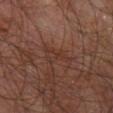Image and clinical context: This image is a 15 mm lesion crop taken from a total-body photograph. The patient is a male approximately 60 years of age. The lesion-visualizer software estimated a border-irregularity rating of about 6.5/10, a color-variation rating of about 2/10, and radial color variation of about 0.5. And it measured a classifier nevus-likeness of about 0/100 and a lesion-detection confidence of about 65/100. This is a cross-polarized tile. The lesion is on the right leg.